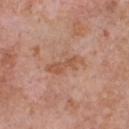follow-up = no biopsy performed (imaged during a skin exam) | lighting = white-light illumination | lesion size = about 4.5 mm | subject = male, aged around 60 | location = the front of the torso | automated metrics = a lesion color around L≈55 a*≈23 b*≈31 in CIELAB, roughly 8 lightness units darker than nearby skin, and a normalized lesion–skin contrast near 6; border irregularity of about 5.5 on a 0–10 scale, a within-lesion color-variation index near 1.5/10, and a peripheral color-asymmetry measure near 0.5 | acquisition = total-body-photography crop, ~15 mm field of view.